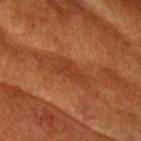<tbp_lesion>
  <biopsy_status>not biopsied; imaged during a skin examination</biopsy_status>
  <patient>
    <sex>female</sex>
    <age_approx>80</age_approx>
  </patient>
  <image>
    <source>total-body photography crop</source>
    <field_of_view_mm>15</field_of_view_mm>
  </image>
  <automated_metrics>
    <border_irregularity_0_10>6.5</border_irregularity_0_10>
    <color_variation_0_10>1.0</color_variation_0_10>
    <peripheral_color_asymmetry>0.0</peripheral_color_asymmetry>
  </automated_metrics>
  <site>head or neck</site>
</tbp_lesion>